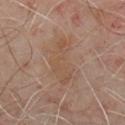The lesion was tiled from a total-body skin photograph and was not biopsied. The lesion is located on the chest. A 15 mm close-up extracted from a 3D total-body photography capture. A male patient aged approximately 50.